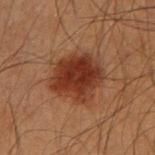Impression: Captured during whole-body skin photography for melanoma surveillance; the lesion was not biopsied. Context: From the arm. Automated tile analysis of the lesion measured roughly 11 lightness units darker than nearby skin. It also reported a nevus-likeness score of about 100/100 and a lesion-detection confidence of about 100/100. Captured under cross-polarized illumination. A male subject, roughly 55 years of age. A close-up tile cropped from a whole-body skin photograph, about 15 mm across.